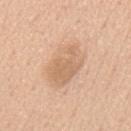Captured during whole-body skin photography for melanoma surveillance; the lesion was not biopsied. A close-up tile cropped from a whole-body skin photograph, about 15 mm across. Automated tile analysis of the lesion measured an average lesion color of about L≈66 a*≈18 b*≈34 (CIELAB). And it measured a border-irregularity index near 2/10, internal color variation of about 3 on a 0–10 scale, and radial color variation of about 1. A male subject, aged 58 to 62. On the mid back. The tile uses white-light illumination. Measured at roughly 6 mm in maximum diameter.